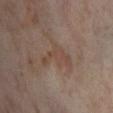Imaged during a routine full-body skin examination; the lesion was not biopsied and no histopathology is available. The lesion is on the left lower leg. A close-up tile cropped from a whole-body skin photograph, about 15 mm across. The lesion-visualizer software estimated a lesion color around L≈45 a*≈16 b*≈25 in CIELAB, about 6 CIELAB-L* units darker than the surrounding skin, and a lesion-to-skin contrast of about 5.5 (normalized; higher = more distinct). It also reported border irregularity of about 8 on a 0–10 scale and internal color variation of about 1.5 on a 0–10 scale. A female subject aged 53–57.